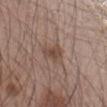biopsy status: no biopsy performed (imaged during a skin exam) | body site: the right forearm | lesion diameter: ~2.5 mm (longest diameter) | patient: male, about 45 years old | lighting: white-light illumination | acquisition: total-body-photography crop, ~15 mm field of view.